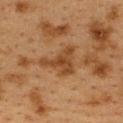biopsy status — total-body-photography surveillance lesion; no biopsy | size — about 4.5 mm | image — ~15 mm crop, total-body skin-cancer survey | body site — the upper back | TBP lesion metrics — a mean CIELAB color near L≈37 a*≈18 b*≈32, a lesion–skin lightness drop of about 8, and a normalized border contrast of about 7.5; a peripheral color-asymmetry measure near 0.5 | patient — female, roughly 40 years of age.